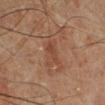Impression: Imaged during a routine full-body skin examination; the lesion was not biopsied and no histopathology is available. Context: Imaged with cross-polarized lighting. A 15 mm close-up tile from a total-body photography series done for melanoma screening. Longest diameter approximately 2.5 mm. A male subject aged approximately 70. Located on the leg.